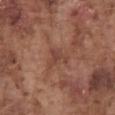{
  "biopsy_status": "not biopsied; imaged during a skin examination",
  "patient": {
    "sex": "male",
    "age_approx": 75
  },
  "image": {
    "source": "total-body photography crop",
    "field_of_view_mm": 15
  },
  "lighting": "white-light",
  "site": "abdomen"
}